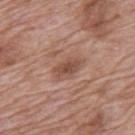Impression:
Part of a total-body skin-imaging series; this lesion was reviewed on a skin check and was not flagged for biopsy.
Background:
About 3 mm across. A male subject approximately 70 years of age. A close-up tile cropped from a whole-body skin photograph, about 15 mm across. The lesion is on the mid back. This is a white-light tile.